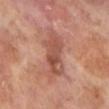Context:
Longest diameter approximately 6.5 mm. A 15 mm close-up tile from a total-body photography series done for melanoma screening. Captured under cross-polarized illumination. A male subject aged 68–72. The lesion is located on the left lower leg.